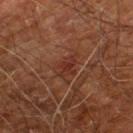Recorded during total-body skin imaging; not selected for excision or biopsy. A male subject aged 58–62. Located on the right leg. A 15 mm close-up tile from a total-body photography series done for melanoma screening.